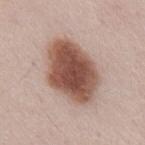{
  "biopsy_status": "not biopsied; imaged during a skin examination",
  "image": {
    "source": "total-body photography crop",
    "field_of_view_mm": 15
  },
  "lesion_size": {
    "long_diameter_mm_approx": 7.5
  },
  "automated_metrics": {
    "area_mm2_approx": 28.0,
    "eccentricity": 0.75,
    "cielab_L": 51,
    "cielab_a": 21,
    "cielab_b": 26,
    "vs_skin_contrast_norm": 12.0
  },
  "site": "abdomen",
  "patient": {
    "sex": "male",
    "age_approx": 50
  }
}